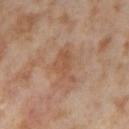body site: the right thigh | subject: female, roughly 55 years of age | image: ~15 mm crop, total-body skin-cancer survey.